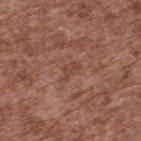  biopsy_status: not biopsied; imaged during a skin examination
  lesion_size:
    long_diameter_mm_approx: 2.5
  automated_metrics:
    area_mm2_approx: 3.0
    eccentricity: 0.85
    shape_asymmetry: 0.55
    cielab_L: 44
    cielab_a: 23
    cielab_b: 28
    vs_skin_contrast_norm: 5.0
    border_irregularity_0_10: 5.0
    color_variation_0_10: 1.0
    peripheral_color_asymmetry: 0.0
    nevus_likeness_0_100: 0
    lesion_detection_confidence_0_100: 100
  site: back
  image:
    source: total-body photography crop
    field_of_view_mm: 15
  patient:
    sex: male
    age_approx: 75